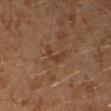biopsy status: catalogued during a skin exam; not biopsied
imaging modality: ~15 mm tile from a whole-body skin photo
diameter: about 3 mm
tile lighting: cross-polarized
location: the left leg
patient: male, approximately 30 years of age